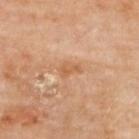biopsy_status: not biopsied; imaged during a skin examination
patient:
  sex: female
  age_approx: 60
image:
  source: total-body photography crop
  field_of_view_mm: 15
lighting: cross-polarized
lesion_size:
  long_diameter_mm_approx: 2.5
site: upper back